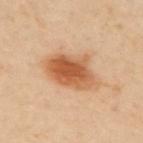Q: Was a biopsy performed?
A: total-body-photography surveillance lesion; no biopsy
Q: What lighting was used for the tile?
A: cross-polarized
Q: How large is the lesion?
A: about 6 mm
Q: What is the imaging modality?
A: ~15 mm crop, total-body skin-cancer survey
Q: Where on the body is the lesion?
A: the upper back
Q: Automated lesion metrics?
A: a lesion area of about 18 mm² and a shape-asymmetry score of about 0.2 (0 = symmetric); a lesion color around L≈58 a*≈24 b*≈38 in CIELAB, about 14 CIELAB-L* units darker than the surrounding skin, and a lesion-to-skin contrast of about 9.5 (normalized; higher = more distinct); a border-irregularity index near 2.5/10, a color-variation rating of about 6.5/10, and a peripheral color-asymmetry measure near 1.5; a classifier nevus-likeness of about 100/100 and a lesion-detection confidence of about 100/100
Q: Who is the patient?
A: male, roughly 65 years of age